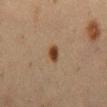Clinical impression: This lesion was catalogued during total-body skin photography and was not selected for biopsy. Image and clinical context: This image is a 15 mm lesion crop taken from a total-body photograph. The lesion is on the abdomen. A male subject aged 58–62.